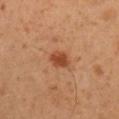The lesion is on the right upper arm.
A male patient aged 53–57.
Cropped from a whole-body photographic skin survey; the tile spans about 15 mm.
Longest diameter approximately 2.5 mm.